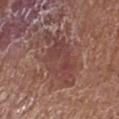  biopsy_status: not biopsied; imaged during a skin examination
  image:
    source: total-body photography crop
    field_of_view_mm: 15
  site: right lower leg
  patient:
    sex: male
    age_approx: 75